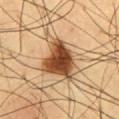{
  "patient": {
    "sex": "male",
    "age_approx": 60
  },
  "image": {
    "source": "total-body photography crop",
    "field_of_view_mm": 15
  },
  "lighting": "cross-polarized",
  "site": "chest"
}